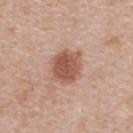Q: Was a biopsy performed?
A: no biopsy performed (imaged during a skin exam)
Q: Lesion size?
A: ≈4 mm
Q: Where on the body is the lesion?
A: the upper back
Q: How was the tile lit?
A: white-light
Q: What kind of image is this?
A: ~15 mm crop, total-body skin-cancer survey
Q: What did automated image analysis measure?
A: an average lesion color of about L≈55 a*≈22 b*≈29 (CIELAB) and a normalized border contrast of about 8.5; border irregularity of about 1.5 on a 0–10 scale, a within-lesion color-variation index near 3.5/10, and radial color variation of about 1; a nevus-likeness score of about 95/100 and lesion-presence confidence of about 100/100
Q: What are the patient's age and sex?
A: female, aged around 45